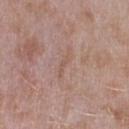Assessment: This lesion was catalogued during total-body skin photography and was not selected for biopsy. Context: Located on the left upper arm. The total-body-photography lesion software estimated a lesion area of about 2 mm², an eccentricity of roughly 0.95, and a symmetry-axis asymmetry near 0.55. And it measured a border-irregularity index near 6/10. The analysis additionally found a classifier nevus-likeness of about 0/100 and lesion-presence confidence of about 80/100. The patient is a male aged approximately 45. A 15 mm close-up tile from a total-body photography series done for melanoma screening.